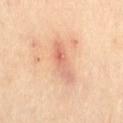{
  "site": "leg",
  "lesion_size": {
    "long_diameter_mm_approx": 5.0
  },
  "image": {
    "source": "total-body photography crop",
    "field_of_view_mm": 15
  },
  "patient": {
    "sex": "female",
    "age_approx": 65
  },
  "automated_metrics": {
    "eccentricity": 0.95,
    "shape_asymmetry": 0.4,
    "cielab_L": 69,
    "cielab_a": 26,
    "cielab_b": 34,
    "vs_skin_darker_L": 10.0,
    "vs_skin_contrast_norm": 6.0,
    "border_irregularity_0_10": 5.0,
    "color_variation_0_10": 4.5,
    "peripheral_color_asymmetry": 1.5
  }
}